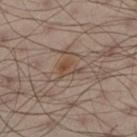Recorded during total-body skin imaging; not selected for excision or biopsy.
A close-up tile cropped from a whole-body skin photograph, about 15 mm across.
A male patient in their 50s.
The lesion is on the left lower leg.
The tile uses cross-polarized illumination.